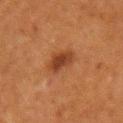Q: Was a biopsy performed?
A: no biopsy performed (imaged during a skin exam)
Q: What kind of image is this?
A: total-body-photography crop, ~15 mm field of view
Q: Who is the patient?
A: male, in their mid- to late 70s
Q: What is the lesion's diameter?
A: about 3 mm
Q: Lesion location?
A: the right upper arm